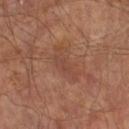follow-up: catalogued during a skin exam; not biopsied | patient: male, aged approximately 65 | site: the right lower leg | TBP lesion metrics: a lesion color around L≈43 a*≈22 b*≈27 in CIELAB, roughly 6 lightness units darker than nearby skin, and a lesion-to-skin contrast of about 4.5 (normalized; higher = more distinct); internal color variation of about 2 on a 0–10 scale and peripheral color asymmetry of about 0.5; a classifier nevus-likeness of about 0/100 and a lesion-detection confidence of about 100/100 | image: ~15 mm tile from a whole-body skin photo | tile lighting: cross-polarized | lesion size: ~5 mm (longest diameter).